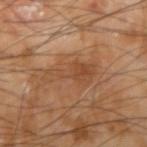Notes:
• biopsy status · total-body-photography surveillance lesion; no biopsy
• image source · total-body-photography crop, ~15 mm field of view
• lighting · cross-polarized
• TBP lesion metrics · a footprint of about 10 mm² and a shape-asymmetry score of about 0.55 (0 = symmetric); about 7 CIELAB-L* units darker than the surrounding skin
• patient · male, in their mid-60s
• anatomic site · the right upper arm